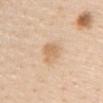{
  "image": {
    "source": "total-body photography crop",
    "field_of_view_mm": 15
  },
  "lesion_size": {
    "long_diameter_mm_approx": 3.5
  },
  "site": "chest",
  "lighting": "white-light",
  "patient": {
    "sex": "male",
    "age_approx": 60
  }
}